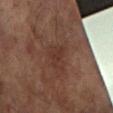Impression:
This lesion was catalogued during total-body skin photography and was not selected for biopsy.
Image and clinical context:
Longest diameter approximately 3 mm. From the left arm. A 15 mm crop from a total-body photograph taken for skin-cancer surveillance. The patient is a female approximately 80 years of age.